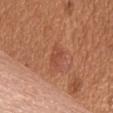biopsy_status: not biopsied; imaged during a skin examination
lesion_size:
  long_diameter_mm_approx: 3.0
automated_metrics:
  area_mm2_approx: 4.0
  eccentricity: 0.85
  shape_asymmetry: 0.3
  border_irregularity_0_10: 3.5
  color_variation_0_10: 1.5
  peripheral_color_asymmetry: 0.5
image:
  source: total-body photography crop
  field_of_view_mm: 15
site: front of the torso
lighting: white-light
patient:
  sex: male
  age_approx: 65